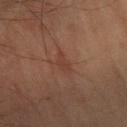Impression: No biopsy was performed on this lesion — it was imaged during a full skin examination and was not determined to be concerning. Clinical summary: An algorithmic analysis of the crop reported a mean CIELAB color near L≈35 a*≈20 b*≈25, a lesion–skin lightness drop of about 5, and a lesion-to-skin contrast of about 5 (normalized; higher = more distinct). This is a cross-polarized tile. The subject is a male aged approximately 60. Measured at roughly 2.5 mm in maximum diameter. Located on the arm. This image is a 15 mm lesion crop taken from a total-body photograph.